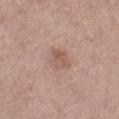Clinical impression:
Recorded during total-body skin imaging; not selected for excision or biopsy.
Background:
A male patient aged 68 to 72. On the leg. Captured under white-light illumination. A region of skin cropped from a whole-body photographic capture, roughly 15 mm wide. About 2.5 mm across.